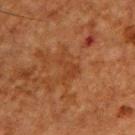Case summary:
* follow-up · total-body-photography surveillance lesion; no biopsy
* automated lesion analysis · an eccentricity of roughly 0.8 and a symmetry-axis asymmetry near 0.5; border irregularity of about 6.5 on a 0–10 scale, a color-variation rating of about 1/10, and a peripheral color-asymmetry measure near 0.5; a classifier nevus-likeness of about 0/100
* image · ~15 mm tile from a whole-body skin photo
* patient · male, roughly 50 years of age
* site · the back